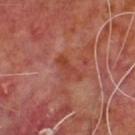<tbp_lesion>
<biopsy_status>not biopsied; imaged during a skin examination</biopsy_status>
<lighting>cross-polarized</lighting>
<site>upper back</site>
<patient>
  <sex>male</sex>
  <age_approx>60</age_approx>
</patient>
<lesion_size>
  <long_diameter_mm_approx>3.5</long_diameter_mm_approx>
</lesion_size>
<automated_metrics>
  <area_mm2_approx>4.5</area_mm2_approx>
  <eccentricity>0.9</eccentricity>
  <shape_asymmetry>0.45</shape_asymmetry>
  <vs_skin_darker_L>6.0</vs_skin_darker_L>
  <vs_skin_contrast_norm>6.0</vs_skin_contrast_norm>
  <peripheral_color_asymmetry>0.5</peripheral_color_asymmetry>
</automated_metrics>
<image>
  <source>total-body photography crop</source>
  <field_of_view_mm>15</field_of_view_mm>
</image>
</tbp_lesion>